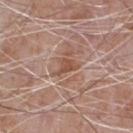patient: male, aged approximately 65 | size: ≈3 mm | acquisition: total-body-photography crop, ~15 mm field of view | anatomic site: the chest.